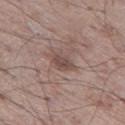Impression: Imaged during a routine full-body skin examination; the lesion was not biopsied and no histopathology is available. Clinical summary: A male patient roughly 50 years of age. A 15 mm close-up extracted from a 3D total-body photography capture. This is a white-light tile. The lesion is on the left thigh. The lesion's longest dimension is about 2.5 mm.